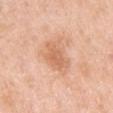{
  "biopsy_status": "not biopsied; imaged during a skin examination",
  "image": {
    "source": "total-body photography crop",
    "field_of_view_mm": 15
  },
  "patient": {
    "sex": "female",
    "age_approx": 50
  },
  "site": "left upper arm"
}